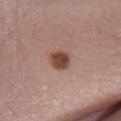{"biopsy_status": "not biopsied; imaged during a skin examination", "lesion_size": {"long_diameter_mm_approx": 2.5}, "site": "leg", "lighting": "white-light", "image": {"source": "total-body photography crop", "field_of_view_mm": 15}, "patient": {"sex": "female", "age_approx": 40}}